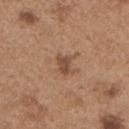Q: Was a biopsy performed?
A: total-body-photography surveillance lesion; no biopsy
Q: Who is the patient?
A: male, aged approximately 65
Q: What is the imaging modality?
A: 15 mm crop, total-body photography
Q: What did automated image analysis measure?
A: a footprint of about 4 mm², a shape eccentricity near 0.7, and two-axis asymmetry of about 0.35; a border-irregularity index near 3.5/10, a within-lesion color-variation index near 1.5/10, and peripheral color asymmetry of about 0.5; a nevus-likeness score of about 20/100
Q: What is the lesion's diameter?
A: about 2.5 mm
Q: Illumination type?
A: white-light
Q: Where on the body is the lesion?
A: the front of the torso